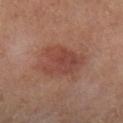Assessment:
The lesion was photographed on a routine skin check and not biopsied; there is no pathology result.
Acquisition and patient details:
Located on the left lower leg. A male subject, aged 63 to 67. Cropped from a total-body skin-imaging series; the visible field is about 15 mm.